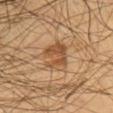Case summary:
– biopsy status — catalogued during a skin exam; not biopsied
– lesion size — ≈4.5 mm
– body site — the chest
– tile lighting — cross-polarized illumination
– patient — male, aged around 55
– automated lesion analysis — an eccentricity of roughly 0.7 and a symmetry-axis asymmetry near 0.3; a border-irregularity index near 4/10, a within-lesion color-variation index near 4/10, and a peripheral color-asymmetry measure near 1.5; an automated nevus-likeness rating near 30 out of 100
– image source — ~15 mm tile from a whole-body skin photo